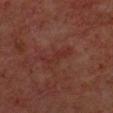Case summary:
– workup — total-body-photography surveillance lesion; no biopsy
– tile lighting — cross-polarized
– image source — ~15 mm tile from a whole-body skin photo
– diameter — ≈4.5 mm
– subject — male, aged 58–62
– location — the upper back